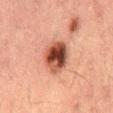Clinical impression:
This lesion was catalogued during total-body skin photography and was not selected for biopsy.
Background:
A male subject approximately 50 years of age. Imaged with cross-polarized lighting. On the mid back. Measured at roughly 4.5 mm in maximum diameter. A 15 mm close-up extracted from a 3D total-body photography capture. The lesion-visualizer software estimated an eccentricity of roughly 0.55 and a symmetry-axis asymmetry near 0.15. And it measured a border-irregularity rating of about 1.5/10, a within-lesion color-variation index near 9.5/10, and a peripheral color-asymmetry measure near 3. The analysis additionally found a classifier nevus-likeness of about 90/100 and a lesion-detection confidence of about 100/100.